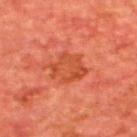  biopsy_status: not biopsied; imaged during a skin examination
  site: back
  patient:
    sex: male
    age_approx: 65
  image:
    source: total-body photography crop
    field_of_view_mm: 15
  lesion_size:
    long_diameter_mm_approx: 4.0
  lighting: cross-polarized